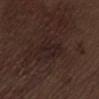Recorded during total-body skin imaging; not selected for excision or biopsy.
Imaged with white-light lighting.
The lesion is on the abdomen.
A male subject aged 68 to 72.
Measured at roughly 3.5 mm in maximum diameter.
The lesion-visualizer software estimated an average lesion color of about L≈20 a*≈14 b*≈15 (CIELAB) and about 4 CIELAB-L* units darker than the surrounding skin. And it measured border irregularity of about 2.5 on a 0–10 scale, a color-variation rating of about 3/10, and radial color variation of about 1.
A roughly 15 mm field-of-view crop from a total-body skin photograph.